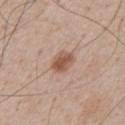Impression: Captured during whole-body skin photography for melanoma surveillance; the lesion was not biopsied. Acquisition and patient details: The lesion-visualizer software estimated an area of roughly 6 mm² and a symmetry-axis asymmetry near 0.2. The analysis additionally found an automated nevus-likeness rating near 90 out of 100 and lesion-presence confidence of about 100/100. The subject is a male in their mid- to late 50s. The recorded lesion diameter is about 3 mm. Cropped from a total-body skin-imaging series; the visible field is about 15 mm. This is a white-light tile.